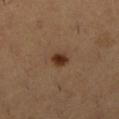| field | value |
|---|---|
| follow-up | total-body-photography surveillance lesion; no biopsy |
| acquisition | total-body-photography crop, ~15 mm field of view |
| tile lighting | cross-polarized illumination |
| anatomic site | the right lower leg |
| patient | female, in their 30s |